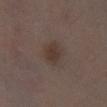Impression:
The lesion was tiled from a total-body skin photograph and was not biopsied.
Acquisition and patient details:
A male patient, aged around 70. A roughly 15 mm field-of-view crop from a total-body skin photograph. The tile uses white-light illumination. An algorithmic analysis of the crop reported an average lesion color of about L≈35 a*≈13 b*≈20 (CIELAB), roughly 7 lightness units darker than nearby skin, and a normalized border contrast of about 6.5. The analysis additionally found a nevus-likeness score of about 40/100 and a detector confidence of about 100 out of 100 that the crop contains a lesion. Approximately 3.5 mm at its widest. Located on the leg.